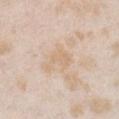notes: total-body-photography surveillance lesion; no biopsy | site: the chest | lesion size: ≈3.5 mm | patient: female, in their mid- to late 20s | image: 15 mm crop, total-body photography.